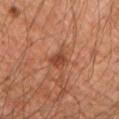Q: Was this lesion biopsied?
A: imaged on a skin check; not biopsied
Q: Patient demographics?
A: male, aged 43 to 47
Q: What kind of image is this?
A: ~15 mm tile from a whole-body skin photo
Q: What did automated image analysis measure?
A: a border-irregularity index near 3/10, internal color variation of about 2.5 on a 0–10 scale, and peripheral color asymmetry of about 1; an automated nevus-likeness rating near 75 out of 100 and a detector confidence of about 100 out of 100 that the crop contains a lesion
Q: Lesion location?
A: the arm